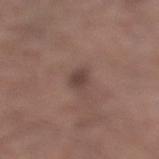Clinical impression:
The lesion was photographed on a routine skin check and not biopsied; there is no pathology result.
Background:
Automated image analysis of the tile measured a footprint of about 3.5 mm² and a shape eccentricity near 0.75. The software also gave a classifier nevus-likeness of about 75/100 and a lesion-detection confidence of about 100/100. A close-up tile cropped from a whole-body skin photograph, about 15 mm across. The lesion's longest dimension is about 2.5 mm. Located on the leg. A male subject in their mid-50s. The tile uses white-light illumination.